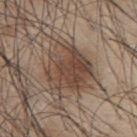This lesion was catalogued during total-body skin photography and was not selected for biopsy. The subject is a male roughly 45 years of age. The lesion is located on the upper back. Measured at roughly 5.5 mm in maximum diameter. A region of skin cropped from a whole-body photographic capture, roughly 15 mm wide.